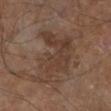The lesion was tiled from a total-body skin photograph and was not biopsied.
A subject roughly 65 years of age.
An algorithmic analysis of the crop reported a footprint of about 23 mm², an eccentricity of roughly 0.75, and two-axis asymmetry of about 0.45. And it measured border irregularity of about 8.5 on a 0–10 scale, internal color variation of about 3 on a 0–10 scale, and a peripheral color-asymmetry measure near 1. And it measured an automated nevus-likeness rating near 0 out of 100 and lesion-presence confidence of about 100/100.
A close-up tile cropped from a whole-body skin photograph, about 15 mm across.
The recorded lesion diameter is about 7 mm.
This is a cross-polarized tile.
The lesion is on the left lower leg.